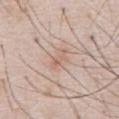No biopsy was performed on this lesion — it was imaged during a full skin examination and was not determined to be concerning. The subject is a male aged 53–57. Cropped from a whole-body photographic skin survey; the tile spans about 15 mm. The lesion is on the chest.